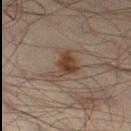Impression: Captured during whole-body skin photography for melanoma surveillance; the lesion was not biopsied. Context: An algorithmic analysis of the crop reported a lesion area of about 8.5 mm² and an outline eccentricity of about 0.7 (0 = round, 1 = elongated). And it measured a lesion color around L≈39 a*≈15 b*≈25 in CIELAB, about 9 CIELAB-L* units darker than the surrounding skin, and a normalized lesion–skin contrast near 9. The software also gave a border-irregularity rating of about 6/10, internal color variation of about 3 on a 0–10 scale, and radial color variation of about 1. And it measured a classifier nevus-likeness of about 90/100 and a lesion-detection confidence of about 100/100. About 4.5 mm across. A male patient aged 63–67. A roughly 15 mm field-of-view crop from a total-body skin photograph. From the leg. This is a cross-polarized tile.